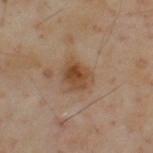<lesion>
<biopsy_status>not biopsied; imaged during a skin examination</biopsy_status>
<lesion_size>
  <long_diameter_mm_approx>3.5</long_diameter_mm_approx>
</lesion_size>
<image>
  <source>total-body photography crop</source>
  <field_of_view_mm>15</field_of_view_mm>
</image>
<site>upper back</site>
<lighting>cross-polarized</lighting>
<patient>
  <sex>male</sex>
  <age_approx>55</age_approx>
</patient>
</lesion>